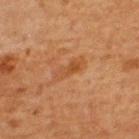The lesion was photographed on a routine skin check and not biopsied; there is no pathology result. The lesion is on the upper back. The subject is a male about 65 years old. Cropped from a whole-body photographic skin survey; the tile spans about 15 mm.